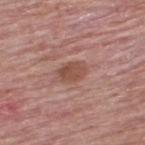The lesion was tiled from a total-body skin photograph and was not biopsied.
Located on the upper back.
A male subject aged 63 to 67.
Longest diameter approximately 3.5 mm.
The tile uses white-light illumination.
A roughly 15 mm field-of-view crop from a total-body skin photograph.